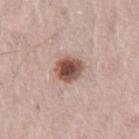Q: Is there a histopathology result?
A: catalogued during a skin exam; not biopsied
Q: What lighting was used for the tile?
A: white-light
Q: What kind of image is this?
A: total-body-photography crop, ~15 mm field of view
Q: Automated lesion metrics?
A: two-axis asymmetry of about 0.15; a color-variation rating of about 6.5/10 and radial color variation of about 1.5; an automated nevus-likeness rating near 100 out of 100 and a detector confidence of about 100 out of 100 that the crop contains a lesion
Q: Lesion location?
A: the leg
Q: What are the patient's age and sex?
A: male, aged 63 to 67
Q: What is the lesion's diameter?
A: ≈3.5 mm